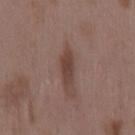Assessment:
Part of a total-body skin-imaging series; this lesion was reviewed on a skin check and was not flagged for biopsy.
Context:
A 15 mm crop from a total-body photograph taken for skin-cancer surveillance. A female subject aged 33–37. The total-body-photography lesion software estimated a footprint of about 5 mm² and an outline eccentricity of about 0.9 (0 = round, 1 = elongated). The analysis additionally found border irregularity of about 3 on a 0–10 scale. The software also gave an automated nevus-likeness rating near 55 out of 100 and lesion-presence confidence of about 100/100. From the mid back.